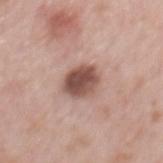Captured during whole-body skin photography for melanoma surveillance; the lesion was not biopsied.
A 15 mm close-up tile from a total-body photography series done for melanoma screening.
Longest diameter approximately 3.5 mm.
The patient is a male approximately 65 years of age.
The lesion is on the mid back.
This is a white-light tile.